The subject is aged approximately 55, imaged with cross-polarized lighting, a 15 mm close-up extracted from a 3D total-body photography capture, from the left forearm, the recorded lesion diameter is about 7 mm:
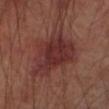histopathologic diagnosis: an actinic keratosis — a borderline lesion of uncertain malignant potential